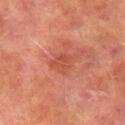biopsy_status: not biopsied; imaged during a skin examination
lesion_size:
  long_diameter_mm_approx: 3.5
patient:
  sex: male
  age_approx: 75
lighting: cross-polarized
site: right lower leg
image:
  source: total-body photography crop
  field_of_view_mm: 15